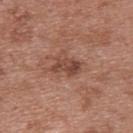workup=imaged on a skin check; not biopsied | TBP lesion metrics=a border-irregularity index near 3.5/10, a within-lesion color-variation index near 4.5/10, and peripheral color asymmetry of about 1.5; a nevus-likeness score of about 0/100 and a detector confidence of about 100 out of 100 that the crop contains a lesion | location=the back | tile lighting=white-light illumination | patient=female, aged 38 to 42 | acquisition=~15 mm crop, total-body skin-cancer survey | lesion size=≈3.5 mm.